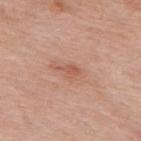workup — no biopsy performed (imaged during a skin exam); body site — the upper back; image source — ~15 mm crop, total-body skin-cancer survey; lesion diameter — ~2.5 mm (longest diameter); tile lighting — white-light; subject — male, aged approximately 55.